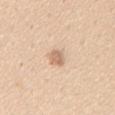| feature | finding |
|---|---|
| automated lesion analysis | roughly 11 lightness units darker than nearby skin and a normalized lesion–skin contrast near 6.5; a nevus-likeness score of about 55/100 and a lesion-detection confidence of about 100/100 |
| subject | male, aged approximately 45 |
| image source | total-body-photography crop, ~15 mm field of view |
| anatomic site | the chest |